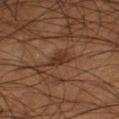biopsy status: imaged on a skin check; not biopsied | automated metrics: an eccentricity of roughly 0.8; a color-variation rating of about 2.5/10; lesion-presence confidence of about 95/100 | image: total-body-photography crop, ~15 mm field of view | patient: male, aged around 55 | tile lighting: cross-polarized | body site: the right lower leg | lesion diameter: about 3.5 mm.